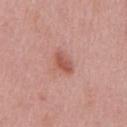Assessment: Part of a total-body skin-imaging series; this lesion was reviewed on a skin check and was not flagged for biopsy. Image and clinical context: Approximately 3 mm at its widest. A roughly 15 mm field-of-view crop from a total-body skin photograph. This is a white-light tile. The total-body-photography lesion software estimated a lesion area of about 5 mm², a shape eccentricity near 0.75, and a shape-asymmetry score of about 0.25 (0 = symmetric). The subject is a male aged 48–52. Located on the chest.